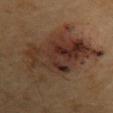Captured under cross-polarized illumination. A close-up tile cropped from a whole-body skin photograph, about 15 mm across. An algorithmic analysis of the crop reported a lesion area of about 41 mm², an eccentricity of roughly 0.85, and a symmetry-axis asymmetry near 0.35. The software also gave a lesion–skin lightness drop of about 9 and a lesion-to-skin contrast of about 9.5 (normalized; higher = more distinct). And it measured a border-irregularity index near 6.5/10, a color-variation rating of about 8.5/10, and radial color variation of about 3. The software also gave a nevus-likeness score of about 30/100. Longest diameter approximately 11 mm. From the chest. The subject is a male aged approximately 70.